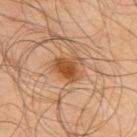• notes: catalogued during a skin exam; not biopsied
• patient: male, about 65 years old
• image: 15 mm crop, total-body photography
• location: the upper back